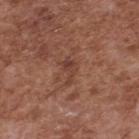Part of a total-body skin-imaging series; this lesion was reviewed on a skin check and was not flagged for biopsy.
A 15 mm close-up extracted from a 3D total-body photography capture.
Captured under white-light illumination.
The lesion is on the back.
The subject is a male aged approximately 75.